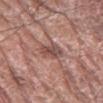Recorded during total-body skin imaging; not selected for excision or biopsy.
This image is a 15 mm lesion crop taken from a total-body photograph.
The lesion-visualizer software estimated a lesion area of about 5.5 mm², an outline eccentricity of about 0.75 (0 = round, 1 = elongated), and a shape-asymmetry score of about 0.25 (0 = symmetric). And it measured internal color variation of about 6 on a 0–10 scale.
Captured under white-light illumination.
A male subject aged approximately 80.
About 3 mm across.
The lesion is located on the right forearm.